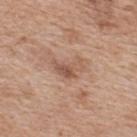notes: catalogued during a skin exam; not biopsied | imaging modality: total-body-photography crop, ~15 mm field of view | body site: the upper back | illumination: white-light illumination | TBP lesion metrics: an average lesion color of about L≈54 a*≈20 b*≈29 (CIELAB), about 9 CIELAB-L* units darker than the surrounding skin, and a lesion-to-skin contrast of about 6.5 (normalized; higher = more distinct); border irregularity of about 6.5 on a 0–10 scale, a color-variation rating of about 3/10, and peripheral color asymmetry of about 1; an automated nevus-likeness rating near 0 out of 100 and a lesion-detection confidence of about 100/100 | patient: female, aged around 40.